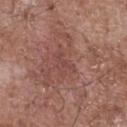{
  "site": "front of the torso",
  "patient": {
    "sex": "male",
    "age_approx": 70
  },
  "image": {
    "source": "total-body photography crop",
    "field_of_view_mm": 15
  },
  "lighting": "white-light",
  "lesion_size": {
    "long_diameter_mm_approx": 3.5
  },
  "automated_metrics": {
    "area_mm2_approx": 6.0,
    "eccentricity": 0.8,
    "border_irregularity_0_10": 4.0,
    "color_variation_0_10": 2.0,
    "peripheral_color_asymmetry": 1.0,
    "nevus_likeness_0_100": 0,
    "lesion_detection_confidence_0_100": 60
  }
}